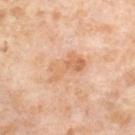workup: total-body-photography surveillance lesion; no biopsy
location: the right thigh
lighting: cross-polarized illumination
subject: female, roughly 55 years of age
imaging modality: ~15 mm tile from a whole-body skin photo